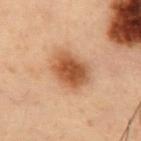{"biopsy_status": "not biopsied; imaged during a skin examination", "site": "chest", "automated_metrics": {"area_mm2_approx": 13.0, "border_irregularity_0_10": 1.5, "peripheral_color_asymmetry": 1.0, "nevus_likeness_0_100": 100, "lesion_detection_confidence_0_100": 100}, "patient": {"sex": "male", "age_approx": 55}, "lighting": "cross-polarized", "lesion_size": {"long_diameter_mm_approx": 4.5}, "image": {"source": "total-body photography crop", "field_of_view_mm": 15}}